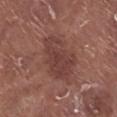Assessment:
Captured during whole-body skin photography for melanoma surveillance; the lesion was not biopsied.
Acquisition and patient details:
A male subject, in their mid-70s. A 15 mm close-up extracted from a 3D total-body photography capture. The lesion is on the right lower leg. An algorithmic analysis of the crop reported an average lesion color of about L≈40 a*≈22 b*≈23 (CIELAB) and roughly 8 lightness units darker than nearby skin. The software also gave a classifier nevus-likeness of about 10/100 and a lesion-detection confidence of about 100/100.